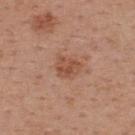workup=catalogued during a skin exam; not biopsied